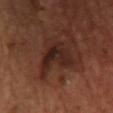Case summary:
– image source · ~15 mm tile from a whole-body skin photo
– site · the chest
– automated lesion analysis · a classifier nevus-likeness of about 25/100 and a detector confidence of about 85 out of 100 that the crop contains a lesion
– lesion size · ≈4 mm
– tile lighting · cross-polarized illumination
– subject · female, about 40 years old